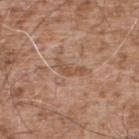No biopsy was performed on this lesion — it was imaged during a full skin examination and was not determined to be concerning. A roughly 15 mm field-of-view crop from a total-body skin photograph. The lesion is located on the back. A male subject aged 53–57.